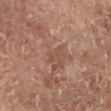<lesion>
  <biopsy_status>not biopsied; imaged during a skin examination</biopsy_status>
  <patient>
    <sex>male</sex>
    <age_approx>65</age_approx>
  </patient>
  <lighting>cross-polarized</lighting>
  <image>
    <source>total-body photography crop</source>
    <field_of_view_mm>15</field_of_view_mm>
  </image>
  <lesion_size>
    <long_diameter_mm_approx>2.5</long_diameter_mm_approx>
  </lesion_size>
  <site>leg</site>
</lesion>